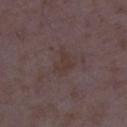Impression:
Captured during whole-body skin photography for melanoma surveillance; the lesion was not biopsied.
Context:
Imaged with white-light lighting. Measured at roughly 3 mm in maximum diameter. Cropped from a whole-body photographic skin survey; the tile spans about 15 mm. A female patient, aged 33–37. The lesion is on the right lower leg.